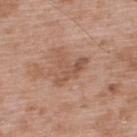biopsy_status: not biopsied; imaged during a skin examination
image:
  source: total-body photography crop
  field_of_view_mm: 15
patient:
  sex: male
  age_approx: 50
site: upper back